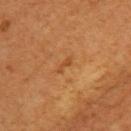Q: Is there a histopathology result?
A: no biopsy performed (imaged during a skin exam)
Q: Patient demographics?
A: female, aged 63 to 67
Q: What is the imaging modality?
A: ~15 mm crop, total-body skin-cancer survey
Q: What did automated image analysis measure?
A: a lesion color around L≈47 a*≈25 b*≈41 in CIELAB and roughly 7 lightness units darker than nearby skin; border irregularity of about 4 on a 0–10 scale and a within-lesion color-variation index near 0/10; a classifier nevus-likeness of about 0/100 and lesion-presence confidence of about 100/100
Q: Illumination type?
A: cross-polarized
Q: Where on the body is the lesion?
A: the left upper arm
Q: Lesion size?
A: about 2.5 mm